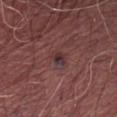Captured during whole-body skin photography for melanoma surveillance; the lesion was not biopsied.
Captured under white-light illumination.
A male subject, in their mid-50s.
A close-up tile cropped from a whole-body skin photograph, about 15 mm across.
The lesion is located on the right thigh.